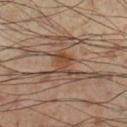Imaged during a routine full-body skin examination; the lesion was not biopsied and no histopathology is available.
The lesion's longest dimension is about 3 mm.
The lesion is located on the leg.
The patient is a male in their mid- to late 50s.
A roughly 15 mm field-of-view crop from a total-body skin photograph.
Captured under cross-polarized illumination.